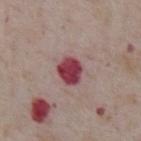Q: Is there a histopathology result?
A: imaged on a skin check; not biopsied
Q: What are the patient's age and sex?
A: male, roughly 75 years of age
Q: How was the tile lit?
A: white-light
Q: Lesion location?
A: the chest
Q: What is the imaging modality?
A: 15 mm crop, total-body photography
Q: Automated lesion metrics?
A: an area of roughly 7.5 mm², an outline eccentricity of about 0.5 (0 = round, 1 = elongated), and two-axis asymmetry of about 0.2; an average lesion color of about L≈42 a*≈31 b*≈17 (CIELAB) and a lesion-to-skin contrast of about 12.5 (normalized; higher = more distinct); a color-variation rating of about 3.5/10 and a peripheral color-asymmetry measure near 1